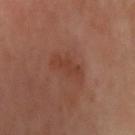Imaged with cross-polarized lighting. An algorithmic analysis of the crop reported an area of roughly 8 mm² and a shape eccentricity near 0.9. The software also gave a color-variation rating of about 2/10 and radial color variation of about 0.5. It also reported a nevus-likeness score of about 0/100 and a lesion-detection confidence of about 100/100. A female patient roughly 60 years of age. A roughly 15 mm field-of-view crop from a total-body skin photograph. From the right upper arm.